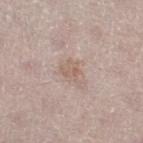workup: catalogued during a skin exam; not biopsied | patient: female, approximately 50 years of age | illumination: white-light | TBP lesion metrics: an area of roughly 4.5 mm² and a symmetry-axis asymmetry near 0.4; an average lesion color of about L≈60 a*≈15 b*≈25 (CIELAB); border irregularity of about 4.5 on a 0–10 scale, internal color variation of about 1.5 on a 0–10 scale, and a peripheral color-asymmetry measure near 0.5; a classifier nevus-likeness of about 0/100 | anatomic site: the right thigh | acquisition: ~15 mm tile from a whole-body skin photo | size: about 3 mm.